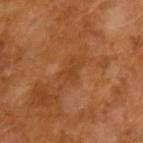notes: catalogued during a skin exam; not biopsied | diameter: ~2.5 mm (longest diameter) | automated metrics: a footprint of about 2.5 mm², a shape eccentricity near 0.85, and two-axis asymmetry of about 0.45; a border-irregularity rating of about 5/10, internal color variation of about 0 on a 0–10 scale, and radial color variation of about 0 | illumination: cross-polarized illumination | subject: male, approximately 65 years of age | image: total-body-photography crop, ~15 mm field of view.